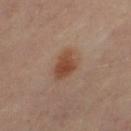{
  "lighting": "cross-polarized",
  "patient": {
    "sex": "female",
    "age_approx": 50
  },
  "site": "right thigh",
  "image": {
    "source": "total-body photography crop",
    "field_of_view_mm": 15
  }
}